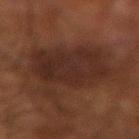Assessment: This lesion was catalogued during total-body skin photography and was not selected for biopsy. Image and clinical context: On the left forearm. A 15 mm close-up tile from a total-body photography series done for melanoma screening. A male subject, aged 63 to 67.